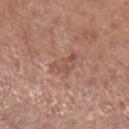Clinical impression: Captured during whole-body skin photography for melanoma surveillance; the lesion was not biopsied. Image and clinical context: A male patient roughly 75 years of age. Located on the left lower leg. Automated image analysis of the tile measured a footprint of about 6 mm² and two-axis asymmetry of about 0.4. The analysis additionally found border irregularity of about 4 on a 0–10 scale. Approximately 3.5 mm at its widest. Imaged with white-light lighting. A 15 mm close-up tile from a total-body photography series done for melanoma screening.